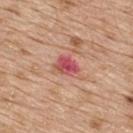Q: Is there a histopathology result?
A: no biopsy performed (imaged during a skin exam)
Q: What is the anatomic site?
A: the upper back
Q: Who is the patient?
A: male, in their 70s
Q: What did automated image analysis measure?
A: an area of roughly 5 mm², an outline eccentricity of about 0.65 (0 = round, 1 = elongated), and a shape-asymmetry score of about 0.25 (0 = symmetric); a border-irregularity rating of about 2.5/10; a classifier nevus-likeness of about 0/100 and lesion-presence confidence of about 100/100
Q: What lighting was used for the tile?
A: white-light illumination
Q: What is the imaging modality?
A: 15 mm crop, total-body photography